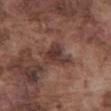workup: no biopsy performed (imaged during a skin exam)
site: the abdomen
image source: 15 mm crop, total-body photography
diameter: ≈3.5 mm
patient: male, aged around 75
tile lighting: white-light